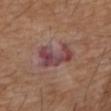Impression:
The lesion was tiled from a total-body skin photograph and was not biopsied.
Image and clinical context:
This is a white-light tile. The patient is a male aged 73 to 77. A lesion tile, about 15 mm wide, cut from a 3D total-body photograph. Located on the back. Measured at roughly 5.5 mm in maximum diameter.